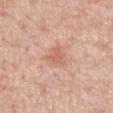| field | value |
|---|---|
| site | the front of the torso |
| patient | male, aged 58–62 |
| lighting | white-light illumination |
| automated lesion analysis | an average lesion color of about L≈63 a*≈25 b*≈30 (CIELAB) and roughly 8 lightness units darker than nearby skin; internal color variation of about 2 on a 0–10 scale; a classifier nevus-likeness of about 10/100 and a lesion-detection confidence of about 100/100 |
| imaging modality | total-body-photography crop, ~15 mm field of view |
| lesion diameter | ≈2.5 mm |